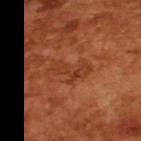Notes:
- biopsy status — catalogued during a skin exam; not biopsied
- imaging modality — 15 mm crop, total-body photography
- lesion size — ~5 mm (longest diameter)
- patient — male, aged 63 to 67